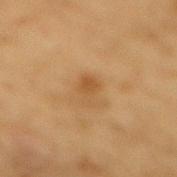This lesion was catalogued during total-body skin photography and was not selected for biopsy. Located on the back. The subject is a male aged approximately 85 or older. The recorded lesion diameter is about 3 mm. Cropped from a total-body skin-imaging series; the visible field is about 15 mm. Captured under cross-polarized illumination.